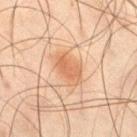Clinical impression:
The lesion was photographed on a routine skin check and not biopsied; there is no pathology result.
Context:
A male patient aged 43 to 47. From the right thigh. Cropped from a total-body skin-imaging series; the visible field is about 15 mm. The tile uses cross-polarized illumination. About 4 mm across.